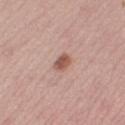Cropped from a total-body skin-imaging series; the visible field is about 15 mm.
On the right thigh.
A female subject, about 25 years old.
The tile uses white-light illumination.